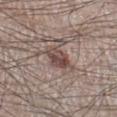Q: Was this lesion biopsied?
A: imaged on a skin check; not biopsied
Q: Who is the patient?
A: male, aged 58 to 62
Q: Illumination type?
A: white-light
Q: What is the anatomic site?
A: the leg
Q: How was this image acquired?
A: total-body-photography crop, ~15 mm field of view
Q: How large is the lesion?
A: about 3 mm